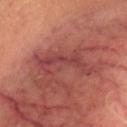  biopsy_status: not biopsied; imaged during a skin examination
  image:
    source: total-body photography crop
    field_of_view_mm: 15
  site: head or neck
  lighting: cross-polarized
  lesion_size:
    long_diameter_mm_approx: 6.0
  patient:
    sex: male
    age_approx: 70
  automated_metrics:
    cielab_L: 42
    cielab_a: 29
    cielab_b: 24
    vs_skin_darker_L: 7.0
    vs_skin_contrast_norm: 6.0
    border_irregularity_0_10: 8.0